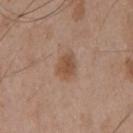Imaged during a routine full-body skin examination; the lesion was not biopsied and no histopathology is available. The lesion's longest dimension is about 3.5 mm. The patient is a male in their mid- to late 50s. From the chest. The tile uses white-light illumination. A region of skin cropped from a whole-body photographic capture, roughly 15 mm wide. Automated image analysis of the tile measured an area of roughly 6.5 mm², a shape eccentricity near 0.65, and a shape-asymmetry score of about 0.2 (0 = symmetric). It also reported roughly 9 lightness units darker than nearby skin and a normalized lesion–skin contrast near 7. And it measured border irregularity of about 2 on a 0–10 scale, internal color variation of about 2.5 on a 0–10 scale, and radial color variation of about 1.